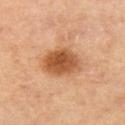biopsy status — imaged on a skin check; not biopsied
site — the left thigh
patient — female, in their mid- to late 50s
lighting — cross-polarized illumination
automated lesion analysis — an average lesion color of about L≈56 a*≈25 b*≈40 (CIELAB), about 15 CIELAB-L* units darker than the surrounding skin, and a lesion-to-skin contrast of about 9.5 (normalized; higher = more distinct); a border-irregularity rating of about 1.5/10, a color-variation rating of about 5/10, and a peripheral color-asymmetry measure near 1.5; a classifier nevus-likeness of about 100/100
image — 15 mm crop, total-body photography
diameter — about 5 mm